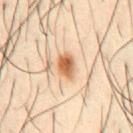Recorded during total-body skin imaging; not selected for excision or biopsy.
A close-up tile cropped from a whole-body skin photograph, about 15 mm across.
The tile uses cross-polarized illumination.
Automated image analysis of the tile measured an area of roughly 5.5 mm² and an outline eccentricity of about 0.75 (0 = round, 1 = elongated). The software also gave internal color variation of about 5.5 on a 0–10 scale.
The lesion is on the abdomen.
The lesion's longest dimension is about 3 mm.
A male patient roughly 40 years of age.